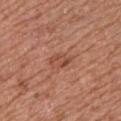Impression:
This lesion was catalogued during total-body skin photography and was not selected for biopsy.
Context:
The lesion is located on the arm. The recorded lesion diameter is about 3 mm. A male subject, aged 73–77. Captured under white-light illumination. A 15 mm crop from a total-body photograph taken for skin-cancer surveillance.